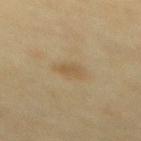The lesion was photographed on a routine skin check and not biopsied; there is no pathology result. A region of skin cropped from a whole-body photographic capture, roughly 15 mm wide. A female patient, aged approximately 60. The lesion is located on the mid back. Imaged with cross-polarized lighting.